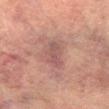Assessment: This lesion was catalogued during total-body skin photography and was not selected for biopsy. Acquisition and patient details: A close-up tile cropped from a whole-body skin photograph, about 15 mm across. The lesion is located on the right lower leg. A male patient aged 73–77.